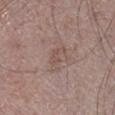Recorded during total-body skin imaging; not selected for excision or biopsy.
About 3.5 mm across.
A male subject, aged around 65.
A lesion tile, about 15 mm wide, cut from a 3D total-body photograph.
On the right lower leg.